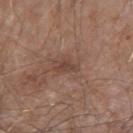| field | value |
|---|---|
| imaging modality | 15 mm crop, total-body photography |
| patient | male, aged 83 or over |
| location | the right upper arm |
| tile lighting | white-light illumination |
| size | ≈3 mm |